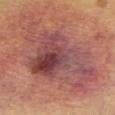This lesion was catalogued during total-body skin photography and was not selected for biopsy. Cropped from a total-body skin-imaging series; the visible field is about 15 mm. A female patient aged 48–52. About 9 mm across. From the chest. The tile uses white-light illumination.